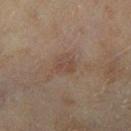The lesion was tiled from a total-body skin photograph and was not biopsied. A female patient, aged 58–62. On the right lower leg. An algorithmic analysis of the crop reported an area of roughly 4.5 mm², an outline eccentricity of about 0.65 (0 = round, 1 = elongated), and a shape-asymmetry score of about 0.2 (0 = symmetric). The software also gave a lesion color around L≈41 a*≈15 b*≈23 in CIELAB, roughly 6 lightness units darker than nearby skin, and a normalized lesion–skin contrast near 5. And it measured a border-irregularity index near 2/10 and a within-lesion color-variation index near 2/10. And it measured a lesion-detection confidence of about 100/100. Cropped from a total-body skin-imaging series; the visible field is about 15 mm. Approximately 2.5 mm at its widest.